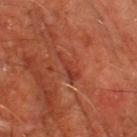| key | value |
|---|---|
| biopsy status | catalogued during a skin exam; not biopsied |
| image source | 15 mm crop, total-body photography |
| tile lighting | cross-polarized illumination |
| anatomic site | the left lower leg |
| lesion size | ≈3.5 mm |
| subject | male, roughly 65 years of age |